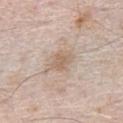Imaged during a routine full-body skin examination; the lesion was not biopsied and no histopathology is available.
A male subject, about 80 years old.
The lesion is located on the chest.
About 3 mm across.
This is a white-light tile.
An algorithmic analysis of the crop reported border irregularity of about 2 on a 0–10 scale, a color-variation rating of about 2/10, and a peripheral color-asymmetry measure near 0.5.
A roughly 15 mm field-of-view crop from a total-body skin photograph.